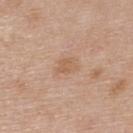| key | value |
|---|---|
| workup | total-body-photography surveillance lesion; no biopsy |
| size | about 2.5 mm |
| lighting | white-light illumination |
| image-analysis metrics | a detector confidence of about 100 out of 100 that the crop contains a lesion |
| subject | female, approximately 65 years of age |
| acquisition | ~15 mm tile from a whole-body skin photo |
| location | the back |